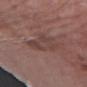This lesion was catalogued during total-body skin photography and was not selected for biopsy. Captured under white-light illumination. The lesion is on the left forearm. The lesion's longest dimension is about 5.5 mm. The patient is a female aged 63–67. The lesion-visualizer software estimated an eccentricity of roughly 0.9. The analysis additionally found a mean CIELAB color near L≈42 a*≈18 b*≈21 and a normalized lesion–skin contrast near 5.5. The analysis additionally found a border-irregularity index near 5/10, a color-variation rating of about 2.5/10, and peripheral color asymmetry of about 0.5. The analysis additionally found lesion-presence confidence of about 90/100. A 15 mm crop from a total-body photograph taken for skin-cancer surveillance.